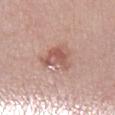Recorded during total-body skin imaging; not selected for excision or biopsy. The patient is a female in their mid-60s. A region of skin cropped from a whole-body photographic capture, roughly 15 mm wide. The lesion's longest dimension is about 3.5 mm. The total-body-photography lesion software estimated border irregularity of about 5 on a 0–10 scale, internal color variation of about 4.5 on a 0–10 scale, and radial color variation of about 1.5. And it measured a classifier nevus-likeness of about 25/100 and a detector confidence of about 100 out of 100 that the crop contains a lesion. Captured under white-light illumination. From the left lower leg.